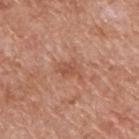Captured during whole-body skin photography for melanoma surveillance; the lesion was not biopsied.
The lesion-visualizer software estimated an automated nevus-likeness rating near 0 out of 100 and a lesion-detection confidence of about 100/100.
A 15 mm close-up tile from a total-body photography series done for melanoma screening.
The subject is a male approximately 60 years of age.
This is a white-light tile.
The lesion is located on the upper back.
Measured at roughly 3 mm in maximum diameter.